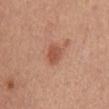Q: Patient demographics?
A: female, in their mid-50s
Q: Automated lesion metrics?
A: a footprint of about 4.5 mm² and a symmetry-axis asymmetry near 0.2; a lesion–skin lightness drop of about 10 and a lesion-to-skin contrast of about 7 (normalized; higher = more distinct); border irregularity of about 1.5 on a 0–10 scale; an automated nevus-likeness rating near 95 out of 100 and lesion-presence confidence of about 100/100
Q: Lesion location?
A: the chest
Q: What kind of image is this?
A: ~15 mm tile from a whole-body skin photo